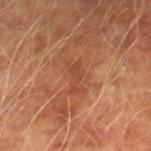{"biopsy_status": "not biopsied; imaged during a skin examination"}